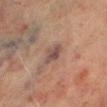Q: Was this lesion biopsied?
A: total-body-photography surveillance lesion; no biopsy
Q: Lesion location?
A: the right lower leg
Q: What is the lesion's diameter?
A: about 3 mm
Q: What are the patient's age and sex?
A: male, roughly 70 years of age
Q: What is the imaging modality?
A: ~15 mm crop, total-body skin-cancer survey
Q: Illumination type?
A: cross-polarized illumination